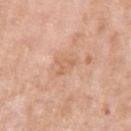Impression: No biopsy was performed on this lesion — it was imaged during a full skin examination and was not determined to be concerning. Image and clinical context: The total-body-photography lesion software estimated a lesion area of about 4 mm² and an outline eccentricity of about 0.5 (0 = round, 1 = elongated). The software also gave a lesion color around L≈64 a*≈21 b*≈34 in CIELAB and a lesion-to-skin contrast of about 4.5 (normalized; higher = more distinct). The analysis additionally found a border-irregularity index near 4.5/10. It also reported a nevus-likeness score of about 0/100. The subject is a female roughly 70 years of age. This is a white-light tile. From the left upper arm. The recorded lesion diameter is about 2.5 mm. Cropped from a whole-body photographic skin survey; the tile spans about 15 mm.